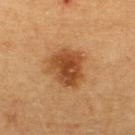Notes:
• follow-up · catalogued during a skin exam; not biopsied
• subject · male, approximately 60 years of age
• location · the upper back
• TBP lesion metrics · an eccentricity of roughly 0.5 and a shape-asymmetry score of about 0.25 (0 = symmetric); a lesion color around L≈42 a*≈22 b*≈36 in CIELAB, roughly 11 lightness units darker than nearby skin, and a lesion-to-skin contrast of about 9 (normalized; higher = more distinct); a classifier nevus-likeness of about 95/100
• illumination · cross-polarized illumination
• lesion size · ~4.5 mm (longest diameter)
• image · ~15 mm crop, total-body skin-cancer survey